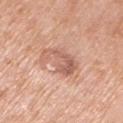Impression:
Part of a total-body skin-imaging series; this lesion was reviewed on a skin check and was not flagged for biopsy.
Acquisition and patient details:
Imaged with white-light lighting. On the left upper arm. Approximately 4.5 mm at its widest. The total-body-photography lesion software estimated a lesion area of about 10 mm², an outline eccentricity of about 0.75 (0 = round, 1 = elongated), and a shape-asymmetry score of about 0.4 (0 = symmetric). And it measured a border-irregularity rating of about 5.5/10, internal color variation of about 4 on a 0–10 scale, and peripheral color asymmetry of about 1. Cropped from a total-body skin-imaging series; the visible field is about 15 mm. A male patient aged 53 to 57.